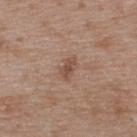Clinical impression: The lesion was photographed on a routine skin check and not biopsied; there is no pathology result. Context: Imaged with white-light lighting. A roughly 15 mm field-of-view crop from a total-body skin photograph. A male subject, roughly 50 years of age. The lesion's longest dimension is about 2.5 mm. From the upper back.